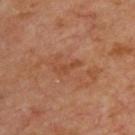Part of a total-body skin-imaging series; this lesion was reviewed on a skin check and was not flagged for biopsy. A roughly 15 mm field-of-view crop from a total-body skin photograph. From the back. A patient about 65 years old. Captured under cross-polarized illumination.